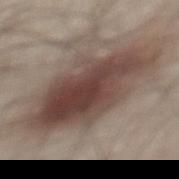  biopsy_status: not biopsied; imaged during a skin examination
  site: mid back
  image:
    source: total-body photography crop
    field_of_view_mm: 15
  patient:
    sex: male
    age_approx: 45
  lesion_size:
    long_diameter_mm_approx: 11.0
  lighting: white-light